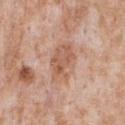Impression:
This lesion was catalogued during total-body skin photography and was not selected for biopsy.
Clinical summary:
The total-body-photography lesion software estimated a lesion area of about 12 mm², an eccentricity of roughly 0.85, and a shape-asymmetry score of about 0.15 (0 = symmetric). Cropped from a whole-body photographic skin survey; the tile spans about 15 mm. About 5 mm across. The lesion is on the chest. The patient is a male aged approximately 65.